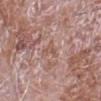The lesion was tiled from a total-body skin photograph and was not biopsied. A roughly 15 mm field-of-view crop from a total-body skin photograph. The subject is a male aged 73 to 77. The lesion is located on the right forearm. The tile uses white-light illumination.